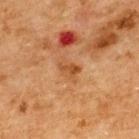Q: Was a biopsy performed?
A: catalogued during a skin exam; not biopsied
Q: What kind of image is this?
A: total-body-photography crop, ~15 mm field of view
Q: What lighting was used for the tile?
A: cross-polarized illumination
Q: Lesion size?
A: ≈3 mm
Q: What are the patient's age and sex?
A: male, in their mid- to late 60s
Q: What is the anatomic site?
A: the upper back